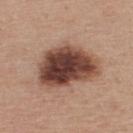Notes:
• workup — imaged on a skin check; not biopsied
• acquisition — ~15 mm crop, total-body skin-cancer survey
• patient — male, approximately 60 years of age
• site — the upper back
• size — ~7.5 mm (longest diameter)
• lighting — white-light illumination